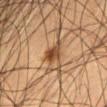Recorded during total-body skin imaging; not selected for excision or biopsy. Longest diameter approximately 2.5 mm. The subject is a male about 50 years old. A roughly 15 mm field-of-view crop from a total-body skin photograph. On the front of the torso.